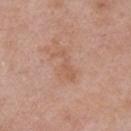Assessment:
This lesion was catalogued during total-body skin photography and was not selected for biopsy.
Image and clinical context:
Automated image analysis of the tile measured a mean CIELAB color near L≈58 a*≈21 b*≈31, a lesion–skin lightness drop of about 6, and a normalized lesion–skin contrast near 5. It also reported border irregularity of about 6.5 on a 0–10 scale, internal color variation of about 1 on a 0–10 scale, and radial color variation of about 0. Approximately 4 mm at its widest. A region of skin cropped from a whole-body photographic capture, roughly 15 mm wide. The tile uses white-light illumination. The patient is a male in their mid- to late 50s. The lesion is located on the right upper arm.